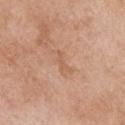Part of a total-body skin-imaging series; this lesion was reviewed on a skin check and was not flagged for biopsy. The subject is a female about 65 years old. Approximately 3 mm at its widest. The lesion is on the chest. A 15 mm crop from a total-body photograph taken for skin-cancer surveillance.